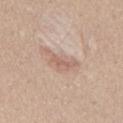biopsy status = catalogued during a skin exam; not biopsied.